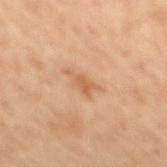Q: Was a biopsy performed?
A: catalogued during a skin exam; not biopsied
Q: How was the tile lit?
A: cross-polarized illumination
Q: What kind of image is this?
A: total-body-photography crop, ~15 mm field of view
Q: What is the anatomic site?
A: the back
Q: Lesion size?
A: ≈3.5 mm
Q: Patient demographics?
A: male, about 60 years old